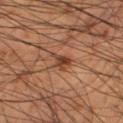Q: Was this lesion biopsied?
A: catalogued during a skin exam; not biopsied
Q: Patient demographics?
A: male, aged approximately 60
Q: What did automated image analysis measure?
A: a shape eccentricity near 0.8 and a symmetry-axis asymmetry near 0.45; radial color variation of about 1.5
Q: Lesion size?
A: about 3.5 mm
Q: Lesion location?
A: the right thigh
Q: Illumination type?
A: cross-polarized
Q: What is the imaging modality?
A: ~15 mm tile from a whole-body skin photo